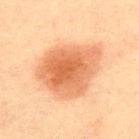Q: Was this lesion biopsied?
A: imaged on a skin check; not biopsied
Q: What did automated image analysis measure?
A: a lesion area of about 34 mm², an outline eccentricity of about 0.6 (0 = round, 1 = elongated), and a symmetry-axis asymmetry near 0.25; an average lesion color of about L≈56 a*≈23 b*≈35 (CIELAB) and about 12 CIELAB-L* units darker than the surrounding skin
Q: Illumination type?
A: cross-polarized
Q: What is the imaging modality?
A: ~15 mm crop, total-body skin-cancer survey
Q: What is the lesion's diameter?
A: ~7.5 mm (longest diameter)
Q: Patient demographics?
A: male, aged 58 to 62
Q: What is the anatomic site?
A: the back